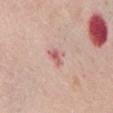Imaged during a routine full-body skin examination; the lesion was not biopsied and no histopathology is available. The subject is a female aged around 75. A 15 mm close-up tile from a total-body photography series done for melanoma screening. The lesion is on the chest. This is a white-light tile. About 2.5 mm across. Automated image analysis of the tile measured a lesion area of about 3.5 mm². The software also gave a border-irregularity index near 4/10, a color-variation rating of about 2.5/10, and a peripheral color-asymmetry measure near 0.5. The analysis additionally found a detector confidence of about 100 out of 100 that the crop contains a lesion.